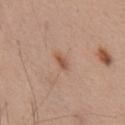The lesion was tiled from a total-body skin photograph and was not biopsied.
A lesion tile, about 15 mm wide, cut from a 3D total-body photograph.
Imaged with white-light lighting.
The patient is a male in their mid-50s.
The lesion is on the upper back.
About 2.5 mm across.
An algorithmic analysis of the crop reported an outline eccentricity of about 0.9 (0 = round, 1 = elongated). The analysis additionally found a lesion color around L≈53 a*≈21 b*≈31 in CIELAB, about 10 CIELAB-L* units darker than the surrounding skin, and a normalized lesion–skin contrast near 7.5. It also reported border irregularity of about 3 on a 0–10 scale and a color-variation rating of about 0/10. It also reported a classifier nevus-likeness of about 60/100.